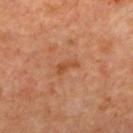Assessment: No biopsy was performed on this lesion — it was imaged during a full skin examination and was not determined to be concerning. Image and clinical context: Cropped from a total-body skin-imaging series; the visible field is about 15 mm. Captured under cross-polarized illumination. The subject is aged 63–67. The lesion's longest dimension is about 3 mm. An algorithmic analysis of the crop reported a lesion color around L≈49 a*≈26 b*≈38 in CIELAB, a lesion–skin lightness drop of about 8, and a normalized border contrast of about 6.5. The software also gave border irregularity of about 4.5 on a 0–10 scale, a within-lesion color-variation index near 0/10, and radial color variation of about 0. And it measured an automated nevus-likeness rating near 0 out of 100. On the upper back.